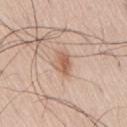biopsy status: imaged on a skin check; not biopsied | location: the lower back | subject: male, aged 58–62 | lesion diameter: ≈2.5 mm | image: 15 mm crop, total-body photography | lighting: white-light.